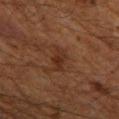The lesion was photographed on a routine skin check and not biopsied; there is no pathology result. Captured under cross-polarized illumination. The patient is a male aged approximately 60. Automated image analysis of the tile measured an outline eccentricity of about 0.9 (0 = round, 1 = elongated) and two-axis asymmetry of about 0.25. And it measured an average lesion color of about L≈24 a*≈17 b*≈24 (CIELAB), roughly 6 lightness units darker than nearby skin, and a normalized lesion–skin contrast near 6.5. It also reported a classifier nevus-likeness of about 5/100 and a lesion-detection confidence of about 100/100. The lesion's longest dimension is about 3.5 mm. From the left thigh. A 15 mm crop from a total-body photograph taken for skin-cancer surveillance.